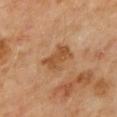No biopsy was performed on this lesion — it was imaged during a full skin examination and was not determined to be concerning.
A male patient roughly 55 years of age.
On the mid back.
A region of skin cropped from a whole-body photographic capture, roughly 15 mm wide.
Imaged with cross-polarized lighting.
Measured at roughly 3.5 mm in maximum diameter.